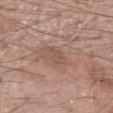Assessment:
The lesion was photographed on a routine skin check and not biopsied; there is no pathology result.
Acquisition and patient details:
Imaged with white-light lighting. Cropped from a whole-body photographic skin survey; the tile spans about 15 mm. A male patient, aged 58–62. Approximately 3 mm at its widest. The lesion is on the arm.